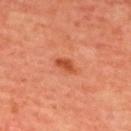The lesion was tiled from a total-body skin photograph and was not biopsied. A male subject roughly 65 years of age. This is a cross-polarized tile. A region of skin cropped from a whole-body photographic capture, roughly 15 mm wide. Located on the upper back. Measured at roughly 3 mm in maximum diameter.